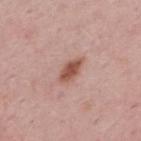Q: Was this lesion biopsied?
A: no biopsy performed (imaged during a skin exam)
Q: What kind of image is this?
A: ~15 mm tile from a whole-body skin photo
Q: Patient demographics?
A: male, roughly 50 years of age
Q: Lesion location?
A: the upper back
Q: Illumination type?
A: white-light
Q: Lesion size?
A: about 3.5 mm